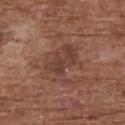Imaged during a routine full-body skin examination; the lesion was not biopsied and no histopathology is available. The lesion is located on the upper back. A close-up tile cropped from a whole-body skin photograph, about 15 mm across. The total-body-photography lesion software estimated a lesion area of about 14 mm², an outline eccentricity of about 0.65 (0 = round, 1 = elongated), and two-axis asymmetry of about 0.4. The analysis additionally found a lesion color around L≈41 a*≈21 b*≈25 in CIELAB, roughly 7 lightness units darker than nearby skin, and a lesion-to-skin contrast of about 6 (normalized; higher = more distinct). It also reported a within-lesion color-variation index near 4/10 and peripheral color asymmetry of about 1.5. The analysis additionally found an automated nevus-likeness rating near 5 out of 100. A female patient aged 73–77. Longest diameter approximately 5 mm. Captured under white-light illumination.